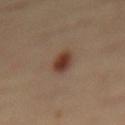This lesion was catalogued during total-body skin photography and was not selected for biopsy.
Cropped from a total-body skin-imaging series; the visible field is about 15 mm.
Located on the mid back.
The patient is a female aged 33 to 37.